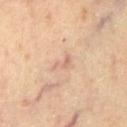<lesion>
  <biopsy_status>not biopsied; imaged during a skin examination</biopsy_status>
  <site>chest</site>
  <image>
    <source>total-body photography crop</source>
    <field_of_view_mm>15</field_of_view_mm>
  </image>
  <lighting>cross-polarized</lighting>
  <automated_metrics>
    <area_mm2_approx>2.5</area_mm2_approx>
    <eccentricity>0.9</eccentricity>
    <shape_asymmetry>0.5</shape_asymmetry>
    <cielab_L>65</cielab_L>
    <cielab_a>20</cielab_a>
    <cielab_b>29</cielab_b>
    <vs_skin_darker_L>8.0</vs_skin_darker_L>
    <vs_skin_contrast_norm>5.0</vs_skin_contrast_norm>
    <border_irregularity_0_10>5.5</border_irregularity_0_10>
    <color_variation_0_10>0.0</color_variation_0_10>
  </automated_metrics>
  <patient>
    <sex>male</sex>
    <age_approx>65</age_approx>
  </patient>
  <lesion_size>
    <long_diameter_mm_approx>3.0</long_diameter_mm_approx>
  </lesion_size>
</lesion>